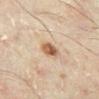biopsy status: catalogued during a skin exam; not biopsied
site: the right lower leg
acquisition: ~15 mm crop, total-body skin-cancer survey
subject: male, in their mid- to late 40s
lighting: cross-polarized illumination